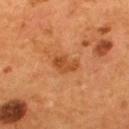body site=the upper back | imaging modality=15 mm crop, total-body photography | lesion size=about 3 mm | subject=male, approximately 55 years of age | lighting=cross-polarized illumination.